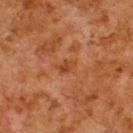Assessment: The lesion was tiled from a total-body skin photograph and was not biopsied. Background: Measured at roughly 2.5 mm in maximum diameter. Imaged with cross-polarized lighting. A 15 mm close-up tile from a total-body photography series done for melanoma screening. The lesion is on the upper back. Automated tile analysis of the lesion measured a shape eccentricity near 0.85 and a symmetry-axis asymmetry near 0.3. A male subject roughly 80 years of age.